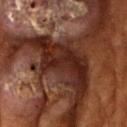Acquisition and patient details: Located on the left upper arm. Captured under cross-polarized illumination. An algorithmic analysis of the crop reported a classifier nevus-likeness of about 0/100. The subject is a female aged 78 to 82. The lesion's longest dimension is about 9.5 mm. This image is a 15 mm lesion crop taken from a total-body photograph.